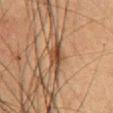The lesion was photographed on a routine skin check and not biopsied; there is no pathology result. A male patient aged around 50. From the chest. A close-up tile cropped from a whole-body skin photograph, about 15 mm across.